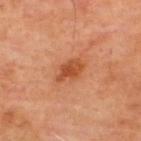Imaged during a routine full-body skin examination; the lesion was not biopsied and no histopathology is available. A region of skin cropped from a whole-body photographic capture, roughly 15 mm wide. About 3.5 mm across. From the upper back. The patient is a male in their mid-60s. The tile uses cross-polarized illumination.